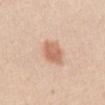<lesion>
<biopsy_status>not biopsied; imaged during a skin examination</biopsy_status>
<site>front of the torso</site>
<patient>
  <sex>female</sex>
  <age_approx>45</age_approx>
</patient>
<image>
  <source>total-body photography crop</source>
  <field_of_view_mm>15</field_of_view_mm>
</image>
<lighting>white-light</lighting>
</lesion>